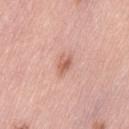<tbp_lesion>
  <biopsy_status>not biopsied; imaged during a skin examination</biopsy_status>
  <lighting>white-light</lighting>
  <patient>
    <sex>female</sex>
    <age_approx>65</age_approx>
  </patient>
  <image>
    <source>total-body photography crop</source>
    <field_of_view_mm>15</field_of_view_mm>
  </image>
  <site>left thigh</site>
</tbp_lesion>